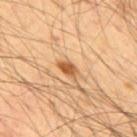Q: Was this lesion biopsied?
A: total-body-photography surveillance lesion; no biopsy
Q: Where on the body is the lesion?
A: the upper back
Q: What is the lesion's diameter?
A: about 2.5 mm
Q: What is the imaging modality?
A: 15 mm crop, total-body photography
Q: How was the tile lit?
A: cross-polarized illumination
Q: Automated lesion metrics?
A: border irregularity of about 2 on a 0–10 scale, a color-variation rating of about 2/10, and a peripheral color-asymmetry measure near 0.5; an automated nevus-likeness rating near 95 out of 100 and a detector confidence of about 100 out of 100 that the crop contains a lesion
Q: Who is the patient?
A: male, aged 53–57